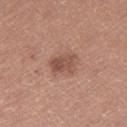Case summary:
• follow-up: catalogued during a skin exam; not biopsied
• lighting: white-light
• TBP lesion metrics: a lesion area of about 5.5 mm², an eccentricity of roughly 0.75, and a shape-asymmetry score of about 0.3 (0 = symmetric); an average lesion color of about L≈50 a*≈22 b*≈26 (CIELAB), a lesion–skin lightness drop of about 10, and a lesion-to-skin contrast of about 7 (normalized; higher = more distinct); an automated nevus-likeness rating near 30 out of 100 and a lesion-detection confidence of about 100/100
• patient: female, roughly 35 years of age
• image: 15 mm crop, total-body photography
• body site: the right thigh
• diameter: about 3 mm